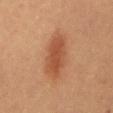Impression:
The lesion was tiled from a total-body skin photograph and was not biopsied.
Image and clinical context:
A female subject roughly 60 years of age. This is a cross-polarized tile. A close-up tile cropped from a whole-body skin photograph, about 15 mm across. The lesion is on the mid back.